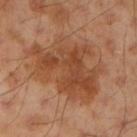Imaged during a routine full-body skin examination; the lesion was not biopsied and no histopathology is available.